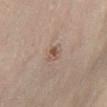Q: Was a biopsy performed?
A: catalogued during a skin exam; not biopsied
Q: What did automated image analysis measure?
A: internal color variation of about 4 on a 0–10 scale and radial color variation of about 1.5; an automated nevus-likeness rating near 15 out of 100 and a lesion-detection confidence of about 100/100
Q: What are the patient's age and sex?
A: male, about 55 years old
Q: Lesion size?
A: ≈2 mm
Q: Lesion location?
A: the left lower leg
Q: How was this image acquired?
A: ~15 mm crop, total-body skin-cancer survey